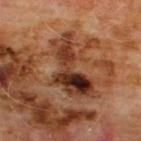patient: male, aged around 60; image source: 15 mm crop, total-body photography; body site: the chest.